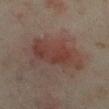Captured during whole-body skin photography for melanoma surveillance; the lesion was not biopsied. The subject is a female roughly 35 years of age. A 15 mm close-up extracted from a 3D total-body photography capture. Located on the left lower leg.